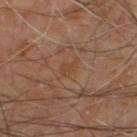Captured during whole-body skin photography for melanoma surveillance; the lesion was not biopsied. The lesion-visualizer software estimated a lesion area of about 3 mm², an eccentricity of roughly 0.8, and two-axis asymmetry of about 0.45. The lesion's longest dimension is about 2.5 mm. A male subject approximately 70 years of age. A 15 mm close-up extracted from a 3D total-body photography capture. The tile uses cross-polarized illumination. From the left lower leg.